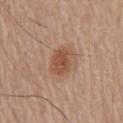Notes:
- notes · total-body-photography surveillance lesion; no biopsy
- image · 15 mm crop, total-body photography
- TBP lesion metrics · a shape eccentricity near 0.65 and two-axis asymmetry of about 0.25; a lesion color around L≈51 a*≈21 b*≈31 in CIELAB, roughly 10 lightness units darker than nearby skin, and a lesion-to-skin contrast of about 7.5 (normalized; higher = more distinct)
- body site · the chest
- subject · male, approximately 65 years of age
- lesion size · about 3.5 mm
- illumination · white-light